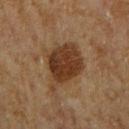follow-up: catalogued during a skin exam; not biopsied